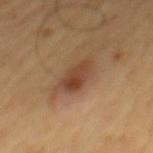subject — male, aged 58 to 62 | acquisition — total-body-photography crop, ~15 mm field of view | location — the mid back.